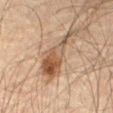Automated image analysis of the tile measured an average lesion color of about L≈40 a*≈14 b*≈25 (CIELAB), about 10 CIELAB-L* units darker than the surrounding skin, and a normalized border contrast of about 8.5. It also reported border irregularity of about 6.5 on a 0–10 scale, a within-lesion color-variation index near 6/10, and peripheral color asymmetry of about 2. The software also gave a nevus-likeness score of about 95/100 and a lesion-detection confidence of about 100/100.
A male patient roughly 60 years of age.
This image is a 15 mm lesion crop taken from a total-body photograph.
Located on the abdomen.
The lesion's longest dimension is about 7 mm.